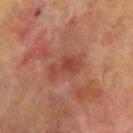The lesion was photographed on a routine skin check and not biopsied; there is no pathology result.
A male patient, roughly 65 years of age.
The lesion is located on the left upper arm.
A 15 mm close-up extracted from a 3D total-body photography capture.